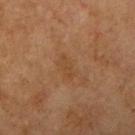Impression:
Imaged during a routine full-body skin examination; the lesion was not biopsied and no histopathology is available.
Context:
The lesion is on the arm. A region of skin cropped from a whole-body photographic capture, roughly 15 mm wide. A female subject, aged 58 to 62.